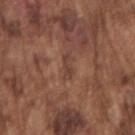follow-up: catalogued during a skin exam; not biopsied | automated lesion analysis: an automated nevus-likeness rating near 0 out of 100 | lesion diameter: ~2.5 mm (longest diameter) | tile lighting: white-light | subject: male, aged around 75 | body site: the arm | acquisition: total-body-photography crop, ~15 mm field of view.